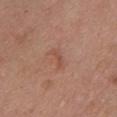{
  "biopsy_status": "not biopsied; imaged during a skin examination",
  "lighting": "white-light",
  "image": {
    "source": "total-body photography crop",
    "field_of_view_mm": 15
  },
  "site": "chest",
  "patient": {
    "sex": "female",
    "age_approx": 50
  },
  "automated_metrics": {
    "area_mm2_approx": 2.5,
    "border_irregularity_0_10": 4.5,
    "color_variation_0_10": 0.0,
    "peripheral_color_asymmetry": 0.0
  },
  "lesion_size": {
    "long_diameter_mm_approx": 2.5
  }
}